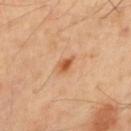biopsy_status: not biopsied; imaged during a skin examination
lesion_size:
  long_diameter_mm_approx: 2.5
automated_metrics:
  eccentricity: 0.8
  shape_asymmetry: 0.35
  border_irregularity_0_10: 3.5
  color_variation_0_10: 3.5
  peripheral_color_asymmetry: 1.0
lighting: cross-polarized
site: mid back
image:
  source: total-body photography crop
  field_of_view_mm: 15
patient:
  sex: male
  age_approx: 40